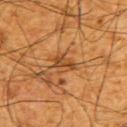Impression: Recorded during total-body skin imaging; not selected for excision or biopsy. Image and clinical context: The recorded lesion diameter is about 3.5 mm. A lesion tile, about 15 mm wide, cut from a 3D total-body photograph. Imaged with cross-polarized lighting. A male patient, about 65 years old. Automated tile analysis of the lesion measured an area of roughly 5 mm², a shape eccentricity near 0.75, and a symmetry-axis asymmetry near 0.35. The lesion is on the upper back.